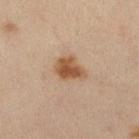This lesion was catalogued during total-body skin photography and was not selected for biopsy. The total-body-photography lesion software estimated a mean CIELAB color near L≈55 a*≈20 b*≈35 and about 12 CIELAB-L* units darker than the surrounding skin. Captured under cross-polarized illumination. A 15 mm close-up extracted from a 3D total-body photography capture. The subject is a female approximately 40 years of age. About 3.5 mm across. The lesion is located on the left leg.